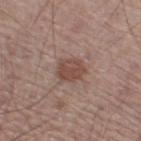The lesion was photographed on a routine skin check and not biopsied; there is no pathology result. The lesion is located on the right thigh. This image is a 15 mm lesion crop taken from a total-body photograph. A male subject in their mid- to late 50s. Imaged with white-light lighting. Approximately 3.5 mm at its widest.